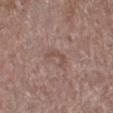Part of a total-body skin-imaging series; this lesion was reviewed on a skin check and was not flagged for biopsy. The lesion is located on the left lower leg. This is a white-light tile. A female patient, roughly 75 years of age. Cropped from a total-body skin-imaging series; the visible field is about 15 mm.